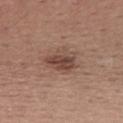Q: Is there a histopathology result?
A: imaged on a skin check; not biopsied
Q: What is the anatomic site?
A: the mid back
Q: What are the patient's age and sex?
A: male, about 40 years old
Q: How was this image acquired?
A: ~15 mm crop, total-body skin-cancer survey